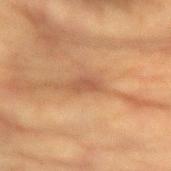biopsy_status: not biopsied; imaged during a skin examination
image:
  source: total-body photography crop
  field_of_view_mm: 15
patient:
  sex: male
  age_approx: 85+
site: right upper arm